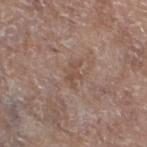Q: Was a biopsy performed?
A: imaged on a skin check; not biopsied
Q: What lighting was used for the tile?
A: white-light illumination
Q: What is the lesion's diameter?
A: ≈3 mm
Q: What is the anatomic site?
A: the left lower leg
Q: Automated lesion metrics?
A: roughly 6 lightness units darker than nearby skin and a lesion-to-skin contrast of about 5 (normalized; higher = more distinct); a border-irregularity rating of about 5/10 and internal color variation of about 1 on a 0–10 scale; a classifier nevus-likeness of about 0/100 and a detector confidence of about 100 out of 100 that the crop contains a lesion
Q: Patient demographics?
A: female, in their mid-70s
Q: How was this image acquired?
A: ~15 mm tile from a whole-body skin photo